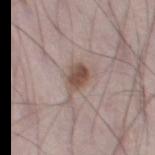{"biopsy_status": "not biopsied; imaged during a skin examination", "site": "left thigh", "automated_metrics": {"area_mm2_approx": 6.0, "shape_asymmetry": 0.25, "color_variation_0_10": 4.0, "peripheral_color_asymmetry": 1.5}, "image": {"source": "total-body photography crop", "field_of_view_mm": 15}, "patient": {"sex": "male", "age_approx": 70}, "lighting": "white-light", "lesion_size": {"long_diameter_mm_approx": 3.5}}